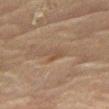Captured under cross-polarized illumination.
From the right thigh.
A female patient in their 80s.
Longest diameter approximately 2.5 mm.
Cropped from a whole-body photographic skin survey; the tile spans about 15 mm.